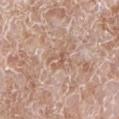follow-up — total-body-photography surveillance lesion; no biopsy